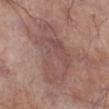{
  "biopsy_status": "not biopsied; imaged during a skin examination",
  "image": {
    "source": "total-body photography crop",
    "field_of_view_mm": 15
  },
  "lighting": "white-light",
  "patient": {
    "sex": "male",
    "age_approx": 70
  },
  "lesion_size": {
    "long_diameter_mm_approx": 8.0
  },
  "site": "right lower leg"
}